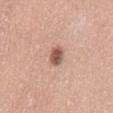A 15 mm close-up tile from a total-body photography series done for melanoma screening.
The lesion is located on the mid back.
A female subject, aged 43–47.